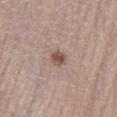Assessment: Captured during whole-body skin photography for melanoma surveillance; the lesion was not biopsied. Image and clinical context: A female subject, approximately 60 years of age. A region of skin cropped from a whole-body photographic capture, roughly 15 mm wide. Located on the leg.